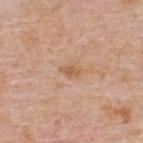<tbp_lesion>
  <biopsy_status>not biopsied; imaged during a skin examination</biopsy_status>
  <site>back</site>
  <lighting>white-light</lighting>
  <patient>
    <sex>male</sex>
    <age_approx>75</age_approx>
  </patient>
  <image>
    <source>total-body photography crop</source>
    <field_of_view_mm>15</field_of_view_mm>
  </image>
  <lesion_size>
    <long_diameter_mm_approx>2.5</long_diameter_mm_approx>
  </lesion_size>
</tbp_lesion>